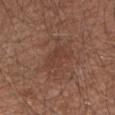Case summary:
• biopsy status — total-body-photography surveillance lesion; no biopsy
• site — the arm
• illumination — white-light illumination
• image — ~15 mm crop, total-body skin-cancer survey
• subject — male, in their mid- to late 50s